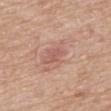{
  "lesion_size": {
    "long_diameter_mm_approx": 2.5
  },
  "automated_metrics": {
    "eccentricity": 0.85,
    "shape_asymmetry": 0.2,
    "border_irregularity_0_10": 2.5,
    "color_variation_0_10": 0.0,
    "peripheral_color_asymmetry": 0.0,
    "nevus_likeness_0_100": 0
  },
  "patient": {
    "sex": "male",
    "age_approx": 80
  },
  "site": "mid back",
  "image": {
    "source": "total-body photography crop",
    "field_of_view_mm": 15
  },
  "lighting": "white-light"
}